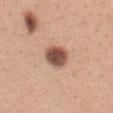<record>
<biopsy_status>not biopsied; imaged during a skin examination</biopsy_status>
<patient>
  <sex>female</sex>
  <age_approx>30</age_approx>
</patient>
<image>
  <source>total-body photography crop</source>
  <field_of_view_mm>15</field_of_view_mm>
</image>
<lesion_size>
  <long_diameter_mm_approx>3.5</long_diameter_mm_approx>
</lesion_size>
<automated_metrics>
  <border_irregularity_0_10>1.0</border_irregularity_0_10>
  <peripheral_color_asymmetry>1.0</peripheral_color_asymmetry>
</automated_metrics>
<lighting>white-light</lighting>
<site>abdomen</site>
</record>